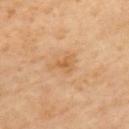Assessment:
Part of a total-body skin-imaging series; this lesion was reviewed on a skin check and was not flagged for biopsy.
Clinical summary:
The lesion is located on the upper back. Automated image analysis of the tile measured an eccentricity of roughly 0.75 and a shape-asymmetry score of about 0.5 (0 = symmetric). It also reported a lesion color around L≈63 a*≈21 b*≈42 in CIELAB, roughly 8 lightness units darker than nearby skin, and a lesion-to-skin contrast of about 6 (normalized; higher = more distinct). It also reported an automated nevus-likeness rating near 0 out of 100 and a lesion-detection confidence of about 100/100. A lesion tile, about 15 mm wide, cut from a 3D total-body photograph. A female subject aged around 65. Longest diameter approximately 3.5 mm. Imaged with cross-polarized lighting.